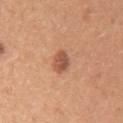Findings:
– biopsy status: imaged on a skin check; not biopsied
– patient: female, approximately 30 years of age
– location: the left upper arm
– illumination: white-light
– image source: total-body-photography crop, ~15 mm field of view
– lesion diameter: ~2.5 mm (longest diameter)